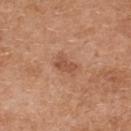notes: imaged on a skin check; not biopsied | anatomic site: the upper back | diameter: ~3 mm (longest diameter) | image source: total-body-photography crop, ~15 mm field of view | lighting: white-light | subject: female, aged approximately 40.